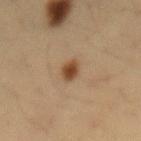notes = catalogued during a skin exam; not biopsied | lighting = cross-polarized illumination | image-analysis metrics = lesion-presence confidence of about 100/100 | site = the lower back | image = 15 mm crop, total-body photography | size = ≈2.5 mm | patient = male, aged approximately 35.